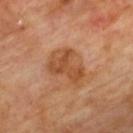automated lesion analysis: a lesion color around L≈48 a*≈23 b*≈36 in CIELAB, roughly 9 lightness units darker than nearby skin, and a lesion-to-skin contrast of about 7.5 (normalized; higher = more distinct); a nevus-likeness score of about 5/100 and a detector confidence of about 100 out of 100 that the crop contains a lesion
image: ~15 mm tile from a whole-body skin photo
subject: male, about 60 years old
site: the upper back
lesion size: ≈4.5 mm
lighting: cross-polarized